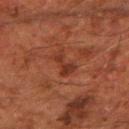The lesion was photographed on a routine skin check and not biopsied; there is no pathology result. From the right forearm. A male patient aged around 60. An algorithmic analysis of the crop reported a shape-asymmetry score of about 0.55 (0 = symmetric). The software also gave a mean CIELAB color near L≈29 a*≈23 b*≈26 and a normalized border contrast of about 7. The analysis additionally found a within-lesion color-variation index near 1.5/10. And it measured an automated nevus-likeness rating near 0 out of 100. Captured under cross-polarized illumination. A 15 mm close-up extracted from a 3D total-body photography capture.